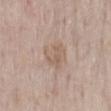Recorded during total-body skin imaging; not selected for excision or biopsy.
Located on the mid back.
This image is a 15 mm lesion crop taken from a total-body photograph.
Automated tile analysis of the lesion measured a lesion–skin lightness drop of about 7. It also reported a border-irregularity rating of about 3/10, a within-lesion color-variation index near 2.5/10, and peripheral color asymmetry of about 1.
A male patient, aged approximately 60.
This is a white-light tile.